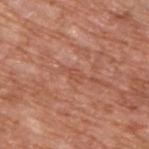biopsy_status: not biopsied; imaged during a skin examination
image:
  source: total-body photography crop
  field_of_view_mm: 15
site: upper back
patient:
  sex: male
  age_approx: 65